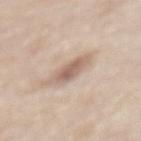Q: Was a biopsy performed?
A: no biopsy performed (imaged during a skin exam)
Q: What kind of image is this?
A: ~15 mm crop, total-body skin-cancer survey
Q: Patient demographics?
A: female, roughly 65 years of age
Q: Lesion location?
A: the mid back